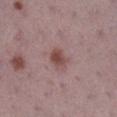  biopsy_status: not biopsied; imaged during a skin examination
  lighting: white-light
  site: right lower leg
  patient:
    sex: female
    age_approx: 40
  image:
    source: total-body photography crop
    field_of_view_mm: 15